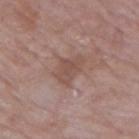Imaged during a routine full-body skin examination; the lesion was not biopsied and no histopathology is available.
Approximately 4 mm at its widest.
The tile uses white-light illumination.
The lesion-visualizer software estimated a symmetry-axis asymmetry near 0.3.
The lesion is located on the right upper arm.
A male subject in their mid- to late 60s.
A close-up tile cropped from a whole-body skin photograph, about 15 mm across.